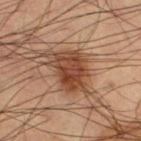Imaged during a routine full-body skin examination; the lesion was not biopsied and no histopathology is available.